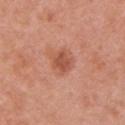Assessment: Part of a total-body skin-imaging series; this lesion was reviewed on a skin check and was not flagged for biopsy. Image and clinical context: About 3 mm across. A female subject approximately 40 years of age. Imaged with white-light lighting. On the arm. Automated tile analysis of the lesion measured a lesion color around L≈53 a*≈27 b*≈32 in CIELAB and about 10 CIELAB-L* units darker than the surrounding skin. A roughly 15 mm field-of-view crop from a total-body skin photograph.